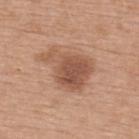biopsy status: total-body-photography surveillance lesion; no biopsy
image: total-body-photography crop, ~15 mm field of view
subject: female, approximately 65 years of age
lesion diameter: about 6 mm
tile lighting: white-light
anatomic site: the upper back